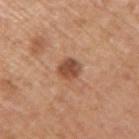Captured during whole-body skin photography for melanoma surveillance; the lesion was not biopsied.
From the right upper arm.
Cropped from a total-body skin-imaging series; the visible field is about 15 mm.
A male subject, in their mid- to late 60s.